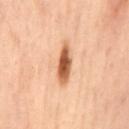| feature | finding |
|---|---|
| biopsy status | imaged on a skin check; not biopsied |
| lesion diameter | ≈5.5 mm |
| illumination | cross-polarized |
| location | the mid back |
| image-analysis metrics | a within-lesion color-variation index near 4.5/10 and peripheral color asymmetry of about 1; a nevus-likeness score of about 100/100 and a detector confidence of about 100 out of 100 that the crop contains a lesion |
| subject | female, aged 53–57 |
| image | 15 mm crop, total-body photography |